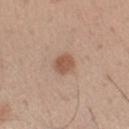Recorded during total-body skin imaging; not selected for excision or biopsy. A roughly 15 mm field-of-view crop from a total-body skin photograph. Longest diameter approximately 2.5 mm. Imaged with white-light lighting. On the left forearm. The subject is a male aged 53–57. An algorithmic analysis of the crop reported an eccentricity of roughly 0.5 and a symmetry-axis asymmetry near 0.2.